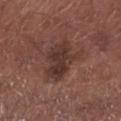This lesion was catalogued during total-body skin photography and was not selected for biopsy.
This image is a 15 mm lesion crop taken from a total-body photograph.
This is a white-light tile.
A male subject aged 53–57.
The lesion-visualizer software estimated a footprint of about 13 mm², a shape eccentricity near 0.6, and a symmetry-axis asymmetry near 0.25.
The lesion is on the left lower leg.